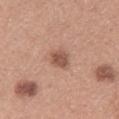The lesion was photographed on a routine skin check and not biopsied; there is no pathology result.
The lesion-visualizer software estimated an average lesion color of about L≈53 a*≈22 b*≈27 (CIELAB), a lesion–skin lightness drop of about 12, and a normalized border contrast of about 8. The analysis additionally found a border-irregularity rating of about 2/10 and a within-lesion color-variation index near 2.5/10.
The subject is a female roughly 30 years of age.
A roughly 15 mm field-of-view crop from a total-body skin photograph.
From the upper back.
Imaged with white-light lighting.
Approximately 2.5 mm at its widest.